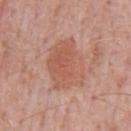<record>
<biopsy_status>not biopsied; imaged during a skin examination</biopsy_status>
<site>front of the torso</site>
<patient>
  <sex>male</sex>
  <age_approx>60</age_approx>
</patient>
<image>
  <source>total-body photography crop</source>
  <field_of_view_mm>15</field_of_view_mm>
</image>
<lesion_size>
  <long_diameter_mm_approx>5.5</long_diameter_mm_approx>
</lesion_size>
<automated_metrics>
  <eccentricity>0.65</eccentricity>
  <cielab_L>57</cielab_L>
  <cielab_a>24</cielab_a>
  <cielab_b>29</cielab_b>
  <border_irregularity_0_10>2.0</border_irregularity_0_10>
  <color_variation_0_10>3.5</color_variation_0_10>
  <peripheral_color_asymmetry>1.0</peripheral_color_asymmetry>
</automated_metrics>
</record>